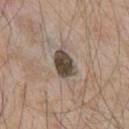biopsy_status: not biopsied; imaged during a skin examination